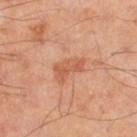Q: Was a biopsy performed?
A: total-body-photography surveillance lesion; no biopsy
Q: Illumination type?
A: cross-polarized illumination
Q: Patient demographics?
A: male, roughly 65 years of age
Q: What did automated image analysis measure?
A: a lesion area of about 6 mm² and a shape-asymmetry score of about 0.4 (0 = symmetric); a lesion color around L≈55 a*≈25 b*≈34 in CIELAB, a lesion–skin lightness drop of about 8, and a lesion-to-skin contrast of about 6 (normalized; higher = more distinct); border irregularity of about 4.5 on a 0–10 scale and internal color variation of about 2 on a 0–10 scale
Q: What kind of image is this?
A: ~15 mm tile from a whole-body skin photo
Q: Lesion location?
A: the left thigh